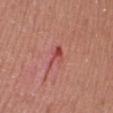Imaged during a routine full-body skin examination; the lesion was not biopsied and no histopathology is available.
The lesion is on the right lower leg.
The patient is a female aged 38–42.
A lesion tile, about 15 mm wide, cut from a 3D total-body photograph.